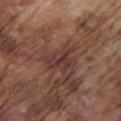This lesion was catalogued during total-body skin photography and was not selected for biopsy.
The tile uses white-light illumination.
Automated tile analysis of the lesion measured a border-irregularity rating of about 4.5/10, internal color variation of about 3.5 on a 0–10 scale, and peripheral color asymmetry of about 1.5. The analysis additionally found a nevus-likeness score of about 0/100 and a detector confidence of about 85 out of 100 that the crop contains a lesion.
A male subject, about 75 years old.
A close-up tile cropped from a whole-body skin photograph, about 15 mm across.
From the left upper arm.